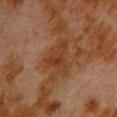tile lighting=cross-polarized illumination
imaging modality=total-body-photography crop, ~15 mm field of view
automated metrics=an eccentricity of roughly 0.75 and two-axis asymmetry of about 0.65; a nevus-likeness score of about 0/100
anatomic site=the front of the torso
lesion size=≈5 mm
patient=male, roughly 80 years of age